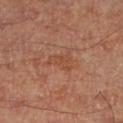follow-up — catalogued during a skin exam; not biopsied | lesion diameter — about 3 mm | site — the right lower leg | lighting — cross-polarized | patient — male, aged approximately 65 | automated lesion analysis — a footprint of about 4 mm², an outline eccentricity of about 0.8 (0 = round, 1 = elongated), and a shape-asymmetry score of about 0.5 (0 = symmetric); a lesion color around L≈47 a*≈24 b*≈32 in CIELAB, roughly 5 lightness units darker than nearby skin, and a normalized border contrast of about 5 | image — ~15 mm crop, total-body skin-cancer survey.